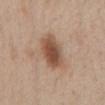No biopsy was performed on this lesion — it was imaged during a full skin examination and was not determined to be concerning. The tile uses white-light illumination. Measured at roughly 4.5 mm in maximum diameter. A lesion tile, about 15 mm wide, cut from a 3D total-body photograph. A male subject, aged 58 to 62. The lesion is located on the front of the torso.